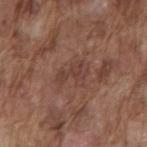follow-up: no biopsy performed (imaged during a skin exam) | image-analysis metrics: an eccentricity of roughly 0.75 and a symmetry-axis asymmetry near 0.5; border irregularity of about 5.5 on a 0–10 scale, a within-lesion color-variation index near 2/10, and a peripheral color-asymmetry measure near 1; a classifier nevus-likeness of about 0/100 | location: the mid back | patient: male, aged approximately 75 | image: ~15 mm crop, total-body skin-cancer survey | lighting: white-light.